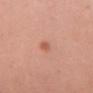| key | value |
|---|---|
| biopsy status | imaged on a skin check; not biopsied |
| image | ~15 mm tile from a whole-body skin photo |
| patient | female, roughly 40 years of age |
| location | the chest |